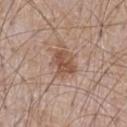<case>
<biopsy_status>not biopsied; imaged during a skin examination</biopsy_status>
<patient>
  <sex>male</sex>
  <age_approx>65</age_approx>
</patient>
<image>
  <source>total-body photography crop</source>
  <field_of_view_mm>15</field_of_view_mm>
</image>
<site>chest</site>
</case>